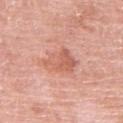Clinical impression: No biopsy was performed on this lesion — it was imaged during a full skin examination and was not determined to be concerning. Image and clinical context: Captured under white-light illumination. The lesion's longest dimension is about 5 mm. A male subject, roughly 80 years of age. The lesion is located on the left upper arm. A region of skin cropped from a whole-body photographic capture, roughly 15 mm wide.